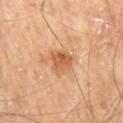The lesion was photographed on a routine skin check and not biopsied; there is no pathology result.